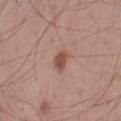Assessment: Recorded during total-body skin imaging; not selected for excision or biopsy. Clinical summary: The total-body-photography lesion software estimated a footprint of about 3.5 mm², an outline eccentricity of about 0.8 (0 = round, 1 = elongated), and a symmetry-axis asymmetry near 0.25. And it measured a mean CIELAB color near L≈50 a*≈22 b*≈27, roughly 12 lightness units darker than nearby skin, and a normalized lesion–skin contrast near 8.5. And it measured lesion-presence confidence of about 100/100. The lesion is on the right thigh. Imaged with white-light lighting. This image is a 15 mm lesion crop taken from a total-body photograph. A male patient aged 58 to 62.